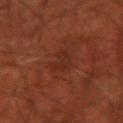| key | value |
|---|---|
| notes | no biopsy performed (imaged during a skin exam) |
| site | the arm |
| image-analysis metrics | a shape eccentricity near 0.85; a lesion color around L≈28 a*≈24 b*≈28 in CIELAB, a lesion–skin lightness drop of about 5, and a normalized lesion–skin contrast near 5; a border-irregularity index near 3.5/10, internal color variation of about 3.5 on a 0–10 scale, and a peripheral color-asymmetry measure near 1 |
| patient | male, aged approximately 70 |
| size | ≈3.5 mm |
| acquisition | ~15 mm crop, total-body skin-cancer survey |